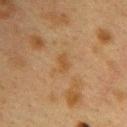Q: Was this lesion biopsied?
A: catalogued during a skin exam; not biopsied
Q: Where on the body is the lesion?
A: the upper back
Q: How large is the lesion?
A: ≈2.5 mm
Q: What are the patient's age and sex?
A: female, aged 38 to 42
Q: What is the imaging modality?
A: ~15 mm crop, total-body skin-cancer survey
Q: What lighting was used for the tile?
A: cross-polarized